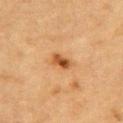{
  "biopsy_status": "not biopsied; imaged during a skin examination",
  "site": "chest",
  "lighting": "cross-polarized",
  "image": {
    "source": "total-body photography crop",
    "field_of_view_mm": 15
  },
  "patient": {
    "sex": "male",
    "age_approx": "85+"
  }
}